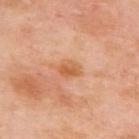No biopsy was performed on this lesion — it was imaged during a full skin examination and was not determined to be concerning. Located on the upper back. The tile uses cross-polarized illumination. The patient is a female about 50 years old. A region of skin cropped from a whole-body photographic capture, roughly 15 mm wide. The lesion's longest dimension is about 2.5 mm.